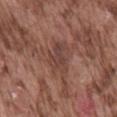follow-up = imaged on a skin check; not biopsied
body site = the back
acquisition = ~15 mm tile from a whole-body skin photo
patient = male, aged 73 to 77